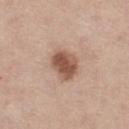Background:
About 3.5 mm across. The total-body-photography lesion software estimated a border-irregularity rating of about 2/10, internal color variation of about 4 on a 0–10 scale, and radial color variation of about 1.5. On the right thigh. Captured under white-light illumination. A 15 mm close-up extracted from a 3D total-body photography capture. A female patient aged 43–47.